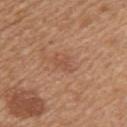Notes:
- notes — imaged on a skin check; not biopsied
- patient — female, aged around 55
- diameter — ≈3 mm
- acquisition — total-body-photography crop, ~15 mm field of view
- illumination — white-light illumination
- site — the left upper arm